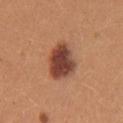Q: Was this lesion biopsied?
A: imaged on a skin check; not biopsied
Q: Where on the body is the lesion?
A: the left upper arm
Q: What is the imaging modality?
A: 15 mm crop, total-body photography
Q: Patient demographics?
A: female, aged around 25
Q: How large is the lesion?
A: ~4 mm (longest diameter)
Q: Automated lesion metrics?
A: an area of roughly 13 mm² and an eccentricity of roughly 0.4; a lesion–skin lightness drop of about 16; a lesion-detection confidence of about 100/100
Q: How was the tile lit?
A: white-light